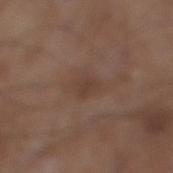Case summary:
- workup: imaged on a skin check; not biopsied